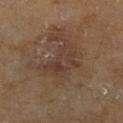| key | value |
|---|---|
| follow-up | no biopsy performed (imaged during a skin exam) |